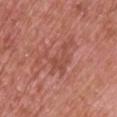Case summary:
– notes: total-body-photography surveillance lesion; no biopsy
– diameter: ~6 mm (longest diameter)
– image source: 15 mm crop, total-body photography
– illumination: white-light illumination
– site: the upper back
– subject: male, in their 70s